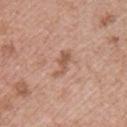| feature | finding |
|---|---|
| biopsy status | catalogued during a skin exam; not biopsied |
| imaging modality | ~15 mm crop, total-body skin-cancer survey |
| image-analysis metrics | a footprint of about 3.5 mm² and a shape eccentricity near 0.9; a mean CIELAB color near L≈55 a*≈21 b*≈30, about 9 CIELAB-L* units darker than the surrounding skin, and a normalized lesion–skin contrast near 6.5; peripheral color asymmetry of about 0 |
| anatomic site | the front of the torso |
| patient | female, aged around 40 |
| lighting | white-light |
| lesion diameter | ~3.5 mm (longest diameter) |